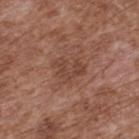Case summary:
* biopsy status: imaged on a skin check; not biopsied
* subject: male, in their mid- to late 70s
* imaging modality: 15 mm crop, total-body photography
* body site: the upper back
* diameter: about 3.5 mm